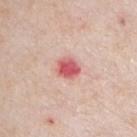biopsy status = catalogued during a skin exam; not biopsied.